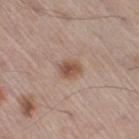The lesion is on the leg. A male subject aged approximately 55. Approximately 2.5 mm at its widest. Cropped from a total-body skin-imaging series; the visible field is about 15 mm. Imaged with white-light lighting. Automated tile analysis of the lesion measured an area of roughly 5 mm² and an eccentricity of roughly 0.6. The software also gave a mean CIELAB color near L≈52 a*≈19 b*≈28, roughly 10 lightness units darker than nearby skin, and a lesion-to-skin contrast of about 8 (normalized; higher = more distinct). And it measured border irregularity of about 1.5 on a 0–10 scale, a color-variation rating of about 3.5/10, and radial color variation of about 1. The software also gave a classifier nevus-likeness of about 95/100 and a lesion-detection confidence of about 100/100.